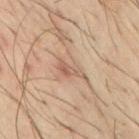Clinical impression: Part of a total-body skin-imaging series; this lesion was reviewed on a skin check and was not flagged for biopsy. Image and clinical context: The patient is a male aged 58–62. Approximately 3.5 mm at its widest. On the mid back. A roughly 15 mm field-of-view crop from a total-body skin photograph.